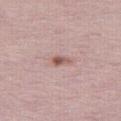| field | value |
|---|---|
| follow-up | no biopsy performed (imaged during a skin exam) |
| automated lesion analysis | a lesion area of about 3 mm², an eccentricity of roughly 0.85, and two-axis asymmetry of about 0.3; a lesion color around L≈56 a*≈20 b*≈23 in CIELAB, roughly 11 lightness units darker than nearby skin, and a normalized border contrast of about 8 |
| lighting | white-light illumination |
| subject | female, in their mid- to late 50s |
| anatomic site | the right thigh |
| imaging modality | 15 mm crop, total-body photography |